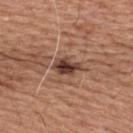Case summary:
• imaging modality · total-body-photography crop, ~15 mm field of view
• size · ~3.5 mm (longest diameter)
• location · the upper back
• patient · male, about 65 years old
• lighting · white-light